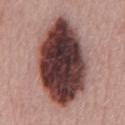image source — ~15 mm crop, total-body skin-cancer survey
illumination — white-light
body site — the front of the torso
subject — male, roughly 75 years of age
size — about 11.5 mm
histopathology — a dysplastic (Clark) nevus